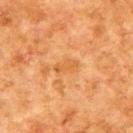notes — no biopsy performed (imaged during a skin exam) | patient — male, aged 78–82 | size — about 3 mm | imaging modality — total-body-photography crop, ~15 mm field of view | lighting — cross-polarized | anatomic site — the upper back.